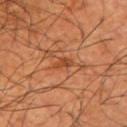Part of a total-body skin-imaging series; this lesion was reviewed on a skin check and was not flagged for biopsy.
From the left upper arm.
This image is a 15 mm lesion crop taken from a total-body photograph.
The total-body-photography lesion software estimated a shape eccentricity near 0.85 and a shape-asymmetry score of about 0.35 (0 = symmetric). And it measured a classifier nevus-likeness of about 5/100 and a lesion-detection confidence of about 95/100.
The subject is a male aged approximately 65.
The recorded lesion diameter is about 2.5 mm.
This is a cross-polarized tile.